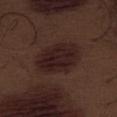No biopsy was performed on this lesion — it was imaged during a full skin examination and was not determined to be concerning. A roughly 15 mm field-of-view crop from a total-body skin photograph. The recorded lesion diameter is about 6 mm. A male subject, aged 68–72. The lesion-visualizer software estimated a footprint of about 18 mm², a shape eccentricity near 0.8, and two-axis asymmetry of about 0.1. The analysis additionally found a lesion–skin lightness drop of about 8 and a lesion-to-skin contrast of about 10 (normalized; higher = more distinct). It also reported a border-irregularity rating of about 1.5/10, a within-lesion color-variation index near 3.5/10, and radial color variation of about 1. It also reported a lesion-detection confidence of about 100/100. Located on the abdomen. Captured under white-light illumination.